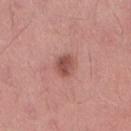Imaged during a routine full-body skin examination; the lesion was not biopsied and no histopathology is available. A lesion tile, about 15 mm wide, cut from a 3D total-body photograph. A male subject, aged around 30. The lesion's longest dimension is about 2.5 mm. The lesion is located on the lower back.